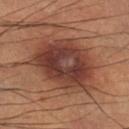biopsy status — catalogued during a skin exam; not biopsied
anatomic site — the right lower leg
patient — male, aged around 50
size — ~7.5 mm (longest diameter)
illumination — cross-polarized illumination
acquisition — ~15 mm tile from a whole-body skin photo